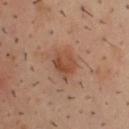A 15 mm crop from a total-body photograph taken for skin-cancer surveillance.
The lesion is located on the upper back.
A male patient aged approximately 40.